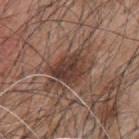Image and clinical context:
Imaged with white-light lighting. Located on the back. The patient is a male aged 43 to 47. A 15 mm crop from a total-body photograph taken for skin-cancer surveillance. Automated image analysis of the tile measured an area of roughly 20 mm², a shape eccentricity near 0.85, and two-axis asymmetry of about 0.4. The analysis additionally found a color-variation rating of about 5.5/10 and a peripheral color-asymmetry measure near 1.5. The analysis additionally found an automated nevus-likeness rating near 80 out of 100 and lesion-presence confidence of about 90/100.